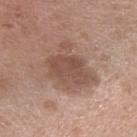No biopsy was performed on this lesion — it was imaged during a full skin examination and was not determined to be concerning. A female subject about 70 years old. A close-up tile cropped from a whole-body skin photograph, about 15 mm across. Automated tile analysis of the lesion measured border irregularity of about 4.5 on a 0–10 scale, internal color variation of about 3.5 on a 0–10 scale, and a peripheral color-asymmetry measure near 1. The tile uses white-light illumination. Located on the right forearm.